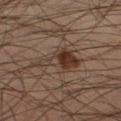biopsy status: total-body-photography surveillance lesion; no biopsy
lesion size: ≈5.5 mm
patient: male, aged 38 to 42
illumination: cross-polarized
image source: ~15 mm crop, total-body skin-cancer survey
anatomic site: the leg